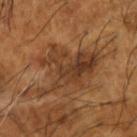Impression: Part of a total-body skin-imaging series; this lesion was reviewed on a skin check and was not flagged for biopsy. Image and clinical context: A male subject, approximately 65 years of age. The lesion is on the left forearm. A region of skin cropped from a whole-body photographic capture, roughly 15 mm wide.